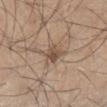{
  "biopsy_status": "not biopsied; imaged during a skin examination",
  "lighting": "white-light",
  "lesion_size": {
    "long_diameter_mm_approx": 2.5
  },
  "automated_metrics": {
    "border_irregularity_0_10": 3.0,
    "color_variation_0_10": 3.5,
    "peripheral_color_asymmetry": 1.0
  },
  "site": "right lower leg",
  "patient": {
    "sex": "male",
    "age_approx": 45
  },
  "image": {
    "source": "total-body photography crop",
    "field_of_view_mm": 15
  }
}